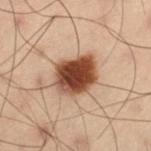biopsy status: total-body-photography surveillance lesion; no biopsy | patient: male, aged around 55 | site: the left thigh | imaging modality: ~15 mm tile from a whole-body skin photo | lesion size: about 5 mm | TBP lesion metrics: a lesion area of about 17 mm², an eccentricity of roughly 0.6, and two-axis asymmetry of about 0.2; a nevus-likeness score of about 100/100 and a detector confidence of about 100 out of 100 that the crop contains a lesion | illumination: cross-polarized.